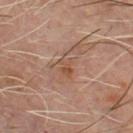| key | value |
|---|---|
| biopsy status | imaged on a skin check; not biopsied |
| body site | the front of the torso |
| tile lighting | cross-polarized illumination |
| TBP lesion metrics | a footprint of about 4.5 mm², an outline eccentricity of about 0.8 (0 = round, 1 = elongated), and a shape-asymmetry score of about 0.3 (0 = symmetric); a border-irregularity rating of about 4.5/10, internal color variation of about 3.5 on a 0–10 scale, and a peripheral color-asymmetry measure near 1 |
| patient | male, approximately 60 years of age |
| acquisition | total-body-photography crop, ~15 mm field of view |
| lesion diameter | ~3 mm (longest diameter) |